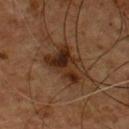Impression: Captured during whole-body skin photography for melanoma surveillance; the lesion was not biopsied. Background: On the chest. A male patient, aged around 55. Cropped from a total-body skin-imaging series; the visible field is about 15 mm.